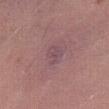workup: catalogued during a skin exam; not biopsied | diameter: ≈2.5 mm | tile lighting: white-light | patient: female, in their mid-30s | anatomic site: the abdomen | image: ~15 mm tile from a whole-body skin photo.